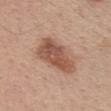Q: Was a biopsy performed?
A: no biopsy performed (imaged during a skin exam)
Q: Lesion location?
A: the head or neck
Q: What is the imaging modality?
A: ~15 mm crop, total-body skin-cancer survey
Q: Who is the patient?
A: female, in their 40s